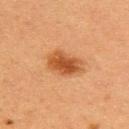Clinical summary:
From the upper back. Cropped from a total-body skin-imaging series; the visible field is about 15 mm. This is a cross-polarized tile. An algorithmic analysis of the crop reported a mean CIELAB color near L≈45 a*≈24 b*≈36 and a normalized border contrast of about 8.5. The software also gave a within-lesion color-variation index near 4/10 and peripheral color asymmetry of about 1. Longest diameter approximately 4.5 mm. The subject is a female in their 40s.